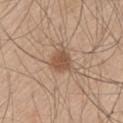{"biopsy_status": "not biopsied; imaged during a skin examination", "image": {"source": "total-body photography crop", "field_of_view_mm": 15}, "site": "left thigh", "patient": {"sex": "male", "age_approx": 60}}